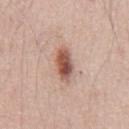Acquisition and patient details: A close-up tile cropped from a whole-body skin photograph, about 15 mm across. This is a white-light tile. The patient is a male in their mid- to late 50s. On the front of the torso. The lesion's longest dimension is about 5 mm.